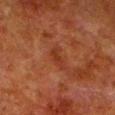| feature | finding |
|---|---|
| workup | imaged on a skin check; not biopsied |
| diameter | about 3 mm |
| image-analysis metrics | an area of roughly 3.5 mm², a shape eccentricity near 0.9, and a symmetry-axis asymmetry near 0.45; a border-irregularity index near 5/10 and a peripheral color-asymmetry measure near 0; lesion-presence confidence of about 100/100 |
| image | ~15 mm tile from a whole-body skin photo |
| body site | the left lower leg |
| subject | male, aged around 80 |
| tile lighting | cross-polarized |